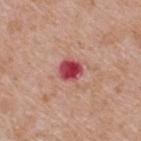| key | value |
|---|---|
| lesion size | ~3 mm (longest diameter) |
| location | the back |
| image | total-body-photography crop, ~15 mm field of view |
| illumination | white-light illumination |
| patient | male, aged 63–67 |